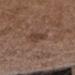Recorded during total-body skin imaging; not selected for excision or biopsy. An algorithmic analysis of the crop reported a border-irregularity index near 3/10, a within-lesion color-variation index near 1.5/10, and radial color variation of about 0.5. The software also gave a nevus-likeness score of about 5/100. From the right forearm. A 15 mm close-up tile from a total-body photography series done for melanoma screening. A female patient in their mid- to late 30s.